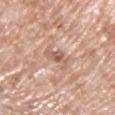This lesion was catalogued during total-body skin photography and was not selected for biopsy. An algorithmic analysis of the crop reported a lesion area of about 4.5 mm², an outline eccentricity of about 0.7 (0 = round, 1 = elongated), and a shape-asymmetry score of about 0.35 (0 = symmetric). And it measured an average lesion color of about L≈59 a*≈19 b*≈28 (CIELAB), roughly 10 lightness units darker than nearby skin, and a normalized border contrast of about 6.5. It also reported a border-irregularity index near 4.5/10, a within-lesion color-variation index near 3/10, and radial color variation of about 0.5. A close-up tile cropped from a whole-body skin photograph, about 15 mm across. The lesion's longest dimension is about 2.5 mm. The lesion is on the left upper arm. The patient is a male aged approximately 70.